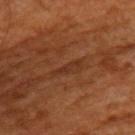Acquisition and patient details:
The lesion is located on the upper back. Imaged with cross-polarized lighting. A 15 mm close-up tile from a total-body photography series done for melanoma screening. The subject is a male aged around 65. Measured at roughly 3.5 mm in maximum diameter.